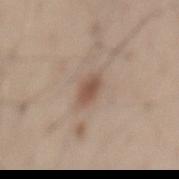Clinical impression:
The lesion was tiled from a total-body skin photograph and was not biopsied.
Context:
The total-body-photography lesion software estimated a lesion color around L≈52 a*≈17 b*≈26 in CIELAB and about 10 CIELAB-L* units darker than the surrounding skin. The analysis additionally found border irregularity of about 2 on a 0–10 scale, a within-lesion color-variation index near 2/10, and peripheral color asymmetry of about 0.5. The analysis additionally found an automated nevus-likeness rating near 80 out of 100 and a detector confidence of about 100 out of 100 that the crop contains a lesion. Captured under white-light illumination. A male patient approximately 55 years of age. The lesion's longest dimension is about 3 mm. A 15 mm close-up extracted from a 3D total-body photography capture. The lesion is on the mid back.